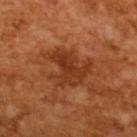biopsy status=imaged on a skin check; not biopsied
image=total-body-photography crop, ~15 mm field of view
illumination=cross-polarized illumination
lesion diameter=about 6 mm
patient=male, aged 63 to 67